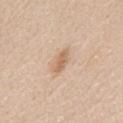follow-up=total-body-photography surveillance lesion; no biopsy
acquisition=~15 mm crop, total-body skin-cancer survey
image-analysis metrics=a lesion color around L≈64 a*≈18 b*≈33 in CIELAB, a lesion–skin lightness drop of about 10, and a normalized lesion–skin contrast near 6.5
size=about 3 mm
location=the mid back
patient=female, aged 58–62